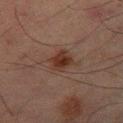- biopsy status · no biopsy performed (imaged during a skin exam)
- diameter · ~3 mm (longest diameter)
- site · the left thigh
- patient · male, aged 63–67
- acquisition · 15 mm crop, total-body photography